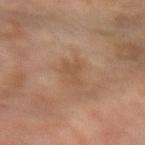biopsy_status: not biopsied; imaged during a skin examination
image:
  source: total-body photography crop
  field_of_view_mm: 15
patient:
  sex: female
  age_approx: 80
site: left forearm